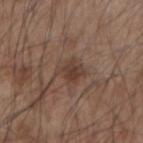Q: Was a biopsy performed?
A: imaged on a skin check; not biopsied
Q: How was this image acquired?
A: ~15 mm crop, total-body skin-cancer survey
Q: Lesion location?
A: the left forearm
Q: Who is the patient?
A: male, about 55 years old